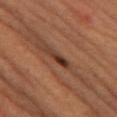workup: total-body-photography surveillance lesion; no biopsy | subject: female, roughly 50 years of age | image-analysis metrics: a shape eccentricity near 0.95 and a symmetry-axis asymmetry near 0.4; a mean CIELAB color near L≈35 a*≈22 b*≈28, a lesion–skin lightness drop of about 12, and a normalized lesion–skin contrast near 10.5; lesion-presence confidence of about 95/100 | acquisition: ~15 mm tile from a whole-body skin photo | illumination: cross-polarized.